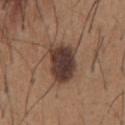Case summary:
* notes · catalogued during a skin exam; not biopsied
* anatomic site · the chest
* automated lesion analysis · a border-irregularity rating of about 2/10 and a within-lesion color-variation index near 5/10; a classifier nevus-likeness of about 45/100
* image · ~15 mm tile from a whole-body skin photo
* size · ≈5 mm
* illumination · white-light illumination
* patient · male, about 65 years old